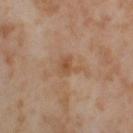  biopsy_status: not biopsied; imaged during a skin examination
  image:
    source: total-body photography crop
    field_of_view_mm: 15
  lighting: cross-polarized
  patient:
    sex: female
    age_approx: 55
  site: right thigh